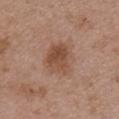biopsy_status: not biopsied; imaged during a skin examination
lighting: white-light
automated_metrics:
  cielab_L: 50
  cielab_a: 20
  cielab_b: 29
  vs_skin_darker_L: 9.0
  vs_skin_contrast_norm: 7.0
  border_irregularity_0_10: 2.0
  color_variation_0_10: 5.0
  peripheral_color_asymmetry: 2.0
site: chest
lesion_size:
  long_diameter_mm_approx: 4.5
patient:
  sex: male
  age_approx: 50
image:
  source: total-body photography crop
  field_of_view_mm: 15